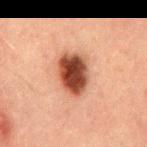biopsy status: total-body-photography surveillance lesion; no biopsy | image source: ~15 mm crop, total-body skin-cancer survey | lighting: cross-polarized illumination | patient: male, aged approximately 50 | site: the mid back | automated lesion analysis: an average lesion color of about L≈37 a*≈22 b*≈27 (CIELAB), roughly 18 lightness units darker than nearby skin, and a lesion-to-skin contrast of about 13.5 (normalized; higher = more distinct); border irregularity of about 1.5 on a 0–10 scale, a within-lesion color-variation index near 6/10, and a peripheral color-asymmetry measure near 1.5; an automated nevus-likeness rating near 100 out of 100 and a detector confidence of about 100 out of 100 that the crop contains a lesion | lesion size: about 5 mm.